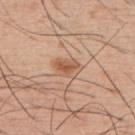The lesion was tiled from a total-body skin photograph and was not biopsied. Approximately 3.5 mm at its widest. Located on the upper back. Cropped from a total-body skin-imaging series; the visible field is about 15 mm. A male patient aged 53 to 57.